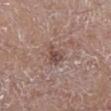biopsy_status: not biopsied; imaged during a skin examination
lesion_size:
  long_diameter_mm_approx: 2.5
site: right lower leg
image:
  source: total-body photography crop
  field_of_view_mm: 15
lighting: white-light
automated_metrics:
  area_mm2_approx: 5.0
  eccentricity: 0.55
  shape_asymmetry: 0.4
  border_irregularity_0_10: 4.5
patient:
  sex: male
  age_approx: 60